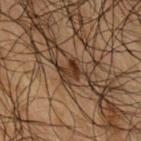No biopsy was performed on this lesion — it was imaged during a full skin examination and was not determined to be concerning. A region of skin cropped from a whole-body photographic capture, roughly 15 mm wide. The lesion is on the arm. A male patient about 50 years old. The lesion-visualizer software estimated an area of roughly 3 mm², a shape eccentricity near 0.75, and a symmetry-axis asymmetry near 0.55. The software also gave a lesion color around L≈25 a*≈14 b*≈23 in CIELAB, about 9 CIELAB-L* units darker than the surrounding skin, and a normalized border contrast of about 9.5. Measured at roughly 2.5 mm in maximum diameter.